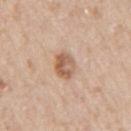Assessment:
Imaged during a routine full-body skin examination; the lesion was not biopsied and no histopathology is available.
Image and clinical context:
On the right upper arm. Imaged with white-light lighting. The lesion's longest dimension is about 3 mm. A lesion tile, about 15 mm wide, cut from a 3D total-body photograph. A female subject aged around 50.